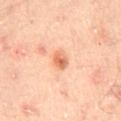Q: Illumination type?
A: cross-polarized illumination
Q: Lesion size?
A: ~2.5 mm (longest diameter)
Q: Lesion location?
A: the back
Q: What is the imaging modality?
A: total-body-photography crop, ~15 mm field of view
Q: Who is the patient?
A: male, aged approximately 65